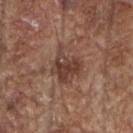lesion size = about 4 mm
imaging modality = 15 mm crop, total-body photography
body site = the head or neck
TBP lesion metrics = an area of roughly 10 mm², an eccentricity of roughly 0.45, and a symmetry-axis asymmetry near 0.35; about 10 CIELAB-L* units darker than the surrounding skin and a normalized border contrast of about 8; a color-variation rating of about 4/10 and radial color variation of about 1.5; an automated nevus-likeness rating near 0 out of 100 and a detector confidence of about 100 out of 100 that the crop contains a lesion
patient = male, aged 78–82
illumination = white-light